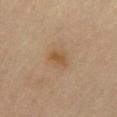Notes:
- workup · total-body-photography surveillance lesion; no biopsy
- location · the front of the torso
- automated metrics · a footprint of about 4.5 mm² and a shape eccentricity near 0.65; an automated nevus-likeness rating near 70 out of 100 and a lesion-detection confidence of about 100/100
- subject · female, roughly 80 years of age
- illumination · cross-polarized illumination
- image source · 15 mm crop, total-body photography
- lesion diameter · ~2.5 mm (longest diameter)